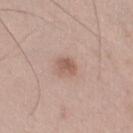Impression:
The lesion was tiled from a total-body skin photograph and was not biopsied.
Clinical summary:
Approximately 2.5 mm at its widest. The lesion is located on the left thigh. Captured under white-light illumination. The patient is a male aged approximately 50. The lesion-visualizer software estimated an outline eccentricity of about 0.65 (0 = round, 1 = elongated) and two-axis asymmetry of about 0.25. And it measured a lesion color around L≈57 a*≈19 b*≈26 in CIELAB, a lesion–skin lightness drop of about 10, and a normalized lesion–skin contrast near 6.5. And it measured an automated nevus-likeness rating near 75 out of 100 and a lesion-detection confidence of about 100/100. Cropped from a whole-body photographic skin survey; the tile spans about 15 mm.